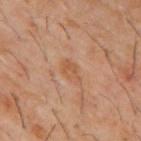Q: Was a biopsy performed?
A: imaged on a skin check; not biopsied
Q: Illumination type?
A: cross-polarized
Q: Patient demographics?
A: male, aged approximately 60
Q: What did automated image analysis measure?
A: an automated nevus-likeness rating near 0 out of 100
Q: How was this image acquired?
A: ~15 mm tile from a whole-body skin photo
Q: Lesion location?
A: the mid back
Q: Lesion size?
A: about 2.5 mm